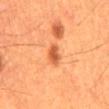| feature | finding |
|---|---|
| biopsy status | total-body-photography surveillance lesion; no biopsy |
| illumination | cross-polarized illumination |
| patient | male, aged around 60 |
| acquisition | ~15 mm crop, total-body skin-cancer survey |
| body site | the mid back |
| size | ~3 mm (longest diameter) |
| image-analysis metrics | an outline eccentricity of about 0.9 (0 = round, 1 = elongated) and two-axis asymmetry of about 0.2 |